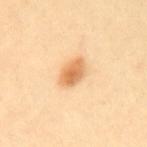Assessment:
Captured during whole-body skin photography for melanoma surveillance; the lesion was not biopsied.
Clinical summary:
A 15 mm crop from a total-body photograph taken for skin-cancer surveillance. On the back. The patient is a female aged around 40. About 3.5 mm across.